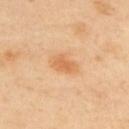<record>
<biopsy_status>not biopsied; imaged during a skin examination</biopsy_status>
<automated_metrics>
  <area_mm2_approx>6.0</area_mm2_approx>
  <eccentricity>0.8</eccentricity>
  <shape_asymmetry>0.25</shape_asymmetry>
  <nevus_likeness_0_100>85</nevus_likeness_0_100>
  <lesion_detection_confidence_0_100>100</lesion_detection_confidence_0_100>
</automated_metrics>
<lesion_size>
  <long_diameter_mm_approx>3.5</long_diameter_mm_approx>
</lesion_size>
<image>
  <source>total-body photography crop</source>
  <field_of_view_mm>15</field_of_view_mm>
</image>
<lighting>cross-polarized</lighting>
<patient>
  <sex>male</sex>
  <age_approx>50</age_approx>
</patient>
<site>upper back</site>
</record>